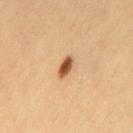biopsy status = total-body-photography surveillance lesion; no biopsy | subject = male, aged 38 to 42 | body site = the left thigh | size = ≈3 mm | acquisition = ~15 mm crop, total-body skin-cancer survey.